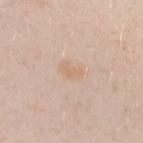biopsy status: catalogued during a skin exam; not biopsied | size: ~3 mm (longest diameter) | imaging modality: 15 mm crop, total-body photography | illumination: white-light | site: the left forearm | automated metrics: a footprint of about 4 mm² and two-axis asymmetry of about 0.3; an automated nevus-likeness rating near 0 out of 100 and a detector confidence of about 100 out of 100 that the crop contains a lesion | subject: female, aged approximately 20.